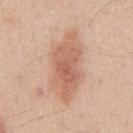Clinical impression:
The lesion was photographed on a routine skin check and not biopsied; there is no pathology result.
Clinical summary:
On the chest. A male subject aged approximately 45. A 15 mm close-up tile from a total-body photography series done for melanoma screening. Captured under white-light illumination. Approximately 8.5 mm at its widest.